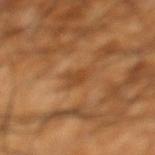Acquisition and patient details: The patient is a male aged 58–62. Located on the left lower leg. A 15 mm close-up tile from a total-body photography series done for melanoma screening.